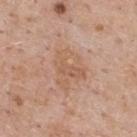| feature | finding |
|---|---|
| illumination | white-light illumination |
| diameter | ≈5 mm |
| image-analysis metrics | an outline eccentricity of about 0.6 (0 = round, 1 = elongated) and a shape-asymmetry score of about 0.35 (0 = symmetric); a color-variation rating of about 4/10 and radial color variation of about 1.5 |
| body site | the upper back |
| subject | male, in their 60s |
| imaging modality | total-body-photography crop, ~15 mm field of view |